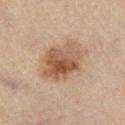follow-up=total-body-photography surveillance lesion; no biopsy | image source=~15 mm crop, total-body skin-cancer survey | location=the right lower leg | image-analysis metrics=a lesion area of about 16 mm² and a shape eccentricity near 0.65; a border-irregularity index near 2.5/10, a color-variation rating of about 6.5/10, and peripheral color asymmetry of about 2 | lesion diameter=~5 mm (longest diameter) | illumination=cross-polarized illumination | patient=male, aged 63–67.